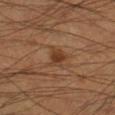Case summary:
• workup — catalogued during a skin exam; not biopsied
• image source — total-body-photography crop, ~15 mm field of view
• body site — the right lower leg
• lesion size — ~2.5 mm (longest diameter)
• subject — male, in their mid-40s
• illumination — cross-polarized illumination